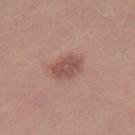Captured during whole-body skin photography for melanoma surveillance; the lesion was not biopsied. A female patient about 20 years old. The lesion-visualizer software estimated a border-irregularity index near 2/10 and internal color variation of about 3 on a 0–10 scale. A roughly 15 mm field-of-view crop from a total-body skin photograph. The recorded lesion diameter is about 3.5 mm. From the right thigh.